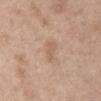  biopsy_status: not biopsied; imaged during a skin examination
  patient:
    sex: female
    age_approx: 40
  site: abdomen
  lesion_size:
    long_diameter_mm_approx: 3.0
  image:
    source: total-body photography crop
    field_of_view_mm: 15